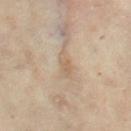This lesion was catalogued during total-body skin photography and was not selected for biopsy.
A 15 mm close-up tile from a total-body photography series done for melanoma screening.
The total-body-photography lesion software estimated a footprint of about 3.5 mm², a shape eccentricity near 0.85, and a symmetry-axis asymmetry near 0.3. And it measured border irregularity of about 3.5 on a 0–10 scale and peripheral color asymmetry of about 0.5.
A female subject, approximately 60 years of age.
Approximately 3 mm at its widest.
On the leg.